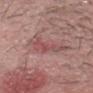lesion size: ~5 mm (longest diameter)
imaging modality: total-body-photography crop, ~15 mm field of view
subject: male, aged around 30
tile lighting: white-light illumination
automated lesion analysis: a lesion color around L≈50 a*≈25 b*≈20 in CIELAB, a lesion–skin lightness drop of about 8, and a lesion-to-skin contrast of about 5.5 (normalized; higher = more distinct); a border-irregularity index near 6.5/10, a within-lesion color-variation index near 1.5/10, and peripheral color asymmetry of about 0